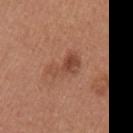Case summary:
- follow-up · imaged on a skin check; not biopsied
- body site · the left upper arm
- lesion size · about 4 mm
- imaging modality · ~15 mm tile from a whole-body skin photo
- patient · female, aged 23–27